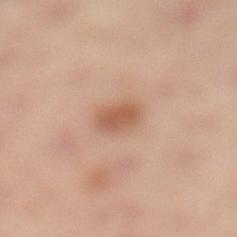Clinical impression: The lesion was tiled from a total-body skin photograph and was not biopsied. Background: A 15 mm crop from a total-body photograph taken for skin-cancer surveillance. From the left lower leg. A female subject aged 53 to 57. This is a cross-polarized tile. The recorded lesion diameter is about 3 mm.